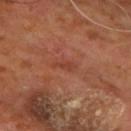The lesion was tiled from a total-body skin photograph and was not biopsied.
The lesion is on the chest.
A male patient roughly 60 years of age.
This image is a 15 mm lesion crop taken from a total-body photograph.